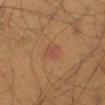Clinical impression:
No biopsy was performed on this lesion — it was imaged during a full skin examination and was not determined to be concerning.
Background:
A region of skin cropped from a whole-body photographic capture, roughly 15 mm wide. The total-body-photography lesion software estimated a border-irregularity index near 2.5/10 and internal color variation of about 2 on a 0–10 scale. A male subject, approximately 65 years of age. Located on the leg.